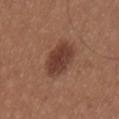<case>
  <biopsy_status>not biopsied; imaged during a skin examination</biopsy_status>
  <lighting>white-light</lighting>
  <automated_metrics>
    <cielab_L>39</cielab_L>
    <cielab_a>22</cielab_a>
    <cielab_b>26</cielab_b>
    <vs_skin_darker_L>11.0</vs_skin_darker_L>
    <vs_skin_contrast_norm>9.5</vs_skin_contrast_norm>
  </automated_metrics>
  <patient>
    <sex>male</sex>
    <age_approx>25</age_approx>
  </patient>
  <lesion_size>
    <long_diameter_mm_approx>4.5</long_diameter_mm_approx>
  </lesion_size>
  <site>chest</site>
  <image>
    <source>total-body photography crop</source>
    <field_of_view_mm>15</field_of_view_mm>
  </image>
</case>